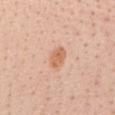{
  "biopsy_status": "not biopsied; imaged during a skin examination",
  "site": "chest",
  "patient": {
    "sex": "female",
    "age_approx": 45
  },
  "image": {
    "source": "total-body photography crop",
    "field_of_view_mm": 15
  }
}